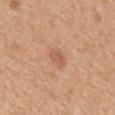Findings:
- workup — imaged on a skin check; not biopsied
- body site — the mid back
- patient — female, in their mid- to late 40s
- image — ~15 mm tile from a whole-body skin photo
- tile lighting — white-light
- lesion diameter — ≈2.5 mm